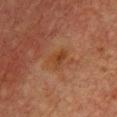This lesion was catalogued during total-body skin photography and was not selected for biopsy. A region of skin cropped from a whole-body photographic capture, roughly 15 mm wide. A female patient aged approximately 50. The lesion is on the chest. The lesion's longest dimension is about 2.5 mm. Automated tile analysis of the lesion measured a mean CIELAB color near L≈35 a*≈21 b*≈32 and about 6 CIELAB-L* units darker than the surrounding skin. And it measured a classifier nevus-likeness of about 0/100 and a detector confidence of about 100 out of 100 that the crop contains a lesion.